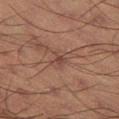The lesion was tiled from a total-body skin photograph and was not biopsied.